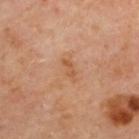Captured during whole-body skin photography for melanoma surveillance; the lesion was not biopsied.
A female patient approximately 60 years of age.
The lesion is on the back.
A 15 mm close-up extracted from a 3D total-body photography capture.